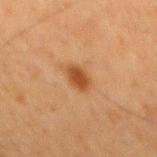The lesion was photographed on a routine skin check and not biopsied; there is no pathology result. The total-body-photography lesion software estimated a footprint of about 4.5 mm² and a shape eccentricity near 0.8. The analysis additionally found a mean CIELAB color near L≈41 a*≈22 b*≈34 and a lesion–skin lightness drop of about 10. It also reported internal color variation of about 2 on a 0–10 scale. And it measured an automated nevus-likeness rating near 95 out of 100 and a detector confidence of about 100 out of 100 that the crop contains a lesion. Captured under cross-polarized illumination. Cropped from a total-body skin-imaging series; the visible field is about 15 mm. The lesion is on the mid back. The subject is a male in their mid-60s. About 3 mm across.